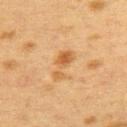biopsy status = no biopsy performed (imaged during a skin exam)
illumination = cross-polarized illumination
lesion size = ~4 mm (longest diameter)
patient = female, aged approximately 40
image source = ~15 mm tile from a whole-body skin photo
location = the back